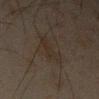- follow-up · total-body-photography surveillance lesion; no biopsy
- lesion size · ≈4.5 mm
- imaging modality · 15 mm crop, total-body photography
- tile lighting · cross-polarized
- patient · male, in their mid- to late 40s
- body site · the right forearm